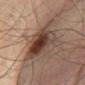Part of a total-body skin-imaging series; this lesion was reviewed on a skin check and was not flagged for biopsy. Approximately 6.5 mm at its widest. The lesion is located on the abdomen. This image is a 15 mm lesion crop taken from a total-body photograph. A male patient, roughly 65 years of age. The tile uses cross-polarized illumination. The total-body-photography lesion software estimated about 12 CIELAB-L* units darker than the surrounding skin and a normalized border contrast of about 9.5. It also reported a within-lesion color-variation index near 9.5/10 and radial color variation of about 3.